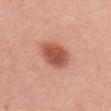Clinical impression:
Part of a total-body skin-imaging series; this lesion was reviewed on a skin check and was not flagged for biopsy.